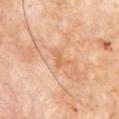Clinical impression:
The lesion was photographed on a routine skin check and not biopsied; there is no pathology result.
Clinical summary:
A male patient about 70 years old. The lesion's longest dimension is about 2.5 mm. From the chest. Imaged with cross-polarized lighting. A 15 mm crop from a total-body photograph taken for skin-cancer surveillance.